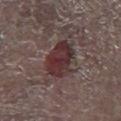Imaged during a routine full-body skin examination; the lesion was not biopsied and no histopathology is available.
A male subject, roughly 70 years of age.
A 15 mm crop from a total-body photograph taken for skin-cancer surveillance.
Approximately 5 mm at its widest.
The tile uses white-light illumination.
On the leg.
The total-body-photography lesion software estimated a lesion area of about 13 mm² and two-axis asymmetry of about 0.2. The software also gave a classifier nevus-likeness of about 75/100 and a lesion-detection confidence of about 100/100.